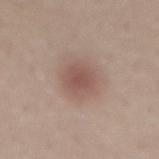Part of a total-body skin-imaging series; this lesion was reviewed on a skin check and was not flagged for biopsy. A region of skin cropped from a whole-body photographic capture, roughly 15 mm wide. A male subject approximately 30 years of age. On the lower back.